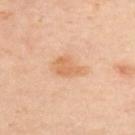biopsy status: catalogued during a skin exam; not biopsied | automated lesion analysis: a shape eccentricity near 0.8; a lesion color around L≈66 a*≈22 b*≈37 in CIELAB and a normalized border contrast of about 6.5 | image source: ~15 mm tile from a whole-body skin photo | site: the upper back | subject: aged 53 to 57.